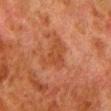<case>
<biopsy_status>not biopsied; imaged during a skin examination</biopsy_status>
<site>leg</site>
<patient>
  <sex>male</sex>
  <age_approx>80</age_approx>
</patient>
<image>
  <source>total-body photography crop</source>
  <field_of_view_mm>15</field_of_view_mm>
</image>
</case>